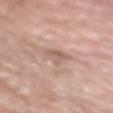* automated lesion analysis — a border-irregularity index near 3.5/10, a within-lesion color-variation index near 3.5/10, and radial color variation of about 1
* lesion size — about 2.5 mm
* site — the right upper arm
* imaging modality — total-body-photography crop, ~15 mm field of view
* subject — male, roughly 75 years of age
* tile lighting — white-light illumination